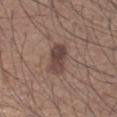Impression:
Captured during whole-body skin photography for melanoma surveillance; the lesion was not biopsied.
Image and clinical context:
The subject is a male roughly 60 years of age. On the arm. Cropped from a total-body skin-imaging series; the visible field is about 15 mm. The recorded lesion diameter is about 4 mm. The tile uses white-light illumination.